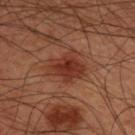- notes · imaged on a skin check; not biopsied
- lighting · cross-polarized illumination
- site · the upper back
- imaging modality · ~15 mm tile from a whole-body skin photo
- patient · male, aged around 45